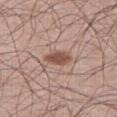Impression:
The lesion was tiled from a total-body skin photograph and was not biopsied.
Clinical summary:
The subject is a male aged 58–62. On the right thigh. A close-up tile cropped from a whole-body skin photograph, about 15 mm across. The lesion's longest dimension is about 3.5 mm.